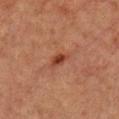The lesion was photographed on a routine skin check and not biopsied; there is no pathology result. A female patient in their 70s. The lesion is located on the front of the torso. About 3 mm across. A roughly 15 mm field-of-view crop from a total-body skin photograph. Imaged with cross-polarized lighting.